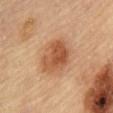Q: Was a biopsy performed?
A: imaged on a skin check; not biopsied